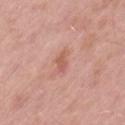| field | value |
|---|---|
| lesion size | about 3 mm |
| location | the left thigh |
| image source | ~15 mm crop, total-body skin-cancer survey |
| TBP lesion metrics | a mean CIELAB color near L≈59 a*≈25 b*≈28 and about 8 CIELAB-L* units darker than the surrounding skin; border irregularity of about 3.5 on a 0–10 scale; a nevus-likeness score of about 0/100 and lesion-presence confidence of about 100/100 |
| patient | male, in their mid- to late 50s |